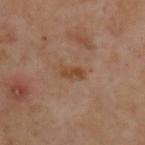Part of a total-body skin-imaging series; this lesion was reviewed on a skin check and was not flagged for biopsy. Measured at roughly 2.5 mm in maximum diameter. This image is a 15 mm lesion crop taken from a total-body photograph. Imaged with cross-polarized lighting. On the upper back. A male subject, aged around 55.